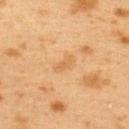Assessment:
The lesion was photographed on a routine skin check and not biopsied; there is no pathology result.
Context:
A female patient, approximately 40 years of age. The total-body-photography lesion software estimated a lesion area of about 3 mm², an outline eccentricity of about 0.9 (0 = round, 1 = elongated), and a symmetry-axis asymmetry near 0.25. The software also gave a mean CIELAB color near L≈52 a*≈19 b*≈37, about 6 CIELAB-L* units darker than the surrounding skin, and a normalized border contrast of about 5. The software also gave border irregularity of about 2.5 on a 0–10 scale, internal color variation of about 0 on a 0–10 scale, and a peripheral color-asymmetry measure near 0. Longest diameter approximately 2.5 mm. A roughly 15 mm field-of-view crop from a total-body skin photograph. The lesion is on the upper back.